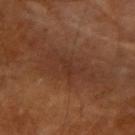biopsy_status: not biopsied; imaged during a skin examination
patient:
  sex: male
  age_approx: 60
automated_metrics:
  area_mm2_approx: 12.0
  eccentricity: 0.9
  color_variation_0_10: 2.5
  peripheral_color_asymmetry: 0.5
  nevus_likeness_0_100: 0
  lesion_detection_confidence_0_100: 60
site: right upper arm
image:
  source: total-body photography crop
  field_of_view_mm: 15
lesion_size:
  long_diameter_mm_approx: 6.0